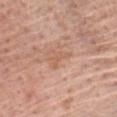<tbp_lesion>
  <biopsy_status>not biopsied; imaged during a skin examination</biopsy_status>
  <lighting>white-light</lighting>
  <image>
    <source>total-body photography crop</source>
    <field_of_view_mm>15</field_of_view_mm>
  </image>
  <patient>
    <sex>male</sex>
    <age_approx>70</age_approx>
  </patient>
  <lesion_size>
    <long_diameter_mm_approx>2.5</long_diameter_mm_approx>
  </lesion_size>
  <automated_metrics>
    <area_mm2_approx>2.5</area_mm2_approx>
    <eccentricity>0.8</eccentricity>
    <shape_asymmetry>0.6</shape_asymmetry>
    <color_variation_0_10>0.0</color_variation_0_10>
    <nevus_likeness_0_100>0</nevus_likeness_0_100>
    <lesion_detection_confidence_0_100>100</lesion_detection_confidence_0_100>
  </automated_metrics>
  <site>chest</site>
</tbp_lesion>